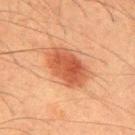Q: Is there a histopathology result?
A: catalogued during a skin exam; not biopsied
Q: What is the anatomic site?
A: the mid back
Q: Illumination type?
A: cross-polarized illumination
Q: Patient demographics?
A: male, approximately 35 years of age
Q: What kind of image is this?
A: ~15 mm crop, total-body skin-cancer survey
Q: How large is the lesion?
A: about 5.5 mm
Q: What did automated image analysis measure?
A: a border-irregularity index near 2/10, internal color variation of about 4 on a 0–10 scale, and radial color variation of about 1.5